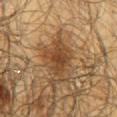{"image": {"source": "total-body photography crop", "field_of_view_mm": 15}, "lighting": "cross-polarized", "patient": {"sex": "male", "age_approx": 65}, "automated_metrics": {"cielab_L": 41, "cielab_a": 19, "cielab_b": 33, "vs_skin_darker_L": 10.0, "vs_skin_contrast_norm": 8.5, "border_irregularity_0_10": 4.5, "color_variation_0_10": 2.5, "peripheral_color_asymmetry": 0.5, "nevus_likeness_0_100": 90, "lesion_detection_confidence_0_100": 100}, "lesion_size": {"long_diameter_mm_approx": 4.0}, "site": "mid back"}